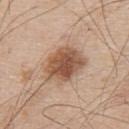{
  "biopsy_status": "not biopsied; imaged during a skin examination",
  "image": {
    "source": "total-body photography crop",
    "field_of_view_mm": 15
  },
  "site": "upper back",
  "lesion_size": {
    "long_diameter_mm_approx": 5.0
  },
  "automated_metrics": {
    "area_mm2_approx": 16.0,
    "eccentricity": 0.6,
    "shape_asymmetry": 0.2,
    "cielab_L": 54,
    "cielab_a": 20,
    "cielab_b": 31,
    "vs_skin_darker_L": 14.0,
    "vs_skin_contrast_norm": 9.5,
    "color_variation_0_10": 5.0,
    "peripheral_color_asymmetry": 1.5,
    "nevus_likeness_0_100": 80,
    "lesion_detection_confidence_0_100": 100
  },
  "patient": {
    "sex": "male",
    "age_approx": 55
  },
  "lighting": "white-light"
}